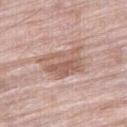Findings:
• follow-up: no biopsy performed (imaged during a skin exam)
• automated metrics: an outline eccentricity of about 0.7 (0 = round, 1 = elongated) and a symmetry-axis asymmetry near 0.3; a border-irregularity index near 3.5/10, internal color variation of about 5.5 on a 0–10 scale, and peripheral color asymmetry of about 2
• site: the leg
• lighting: white-light illumination
• image source: 15 mm crop, total-body photography
• patient: male, aged 78–82